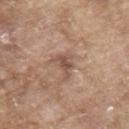The lesion was tiled from a total-body skin photograph and was not biopsied.
On the upper back.
Imaged with white-light lighting.
The patient is a male aged 63–67.
A close-up tile cropped from a whole-body skin photograph, about 15 mm across.
Approximately 3 mm at its widest.